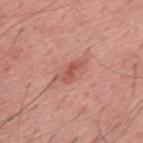Image and clinical context:
About 3 mm across. The subject is a male aged approximately 55. The lesion is on the back. Automated image analysis of the tile measured an area of roughly 3.5 mm², an outline eccentricity of about 0.9 (0 = round, 1 = elongated), and a symmetry-axis asymmetry near 0.35. This is a white-light tile. A region of skin cropped from a whole-body photographic capture, roughly 15 mm wide.